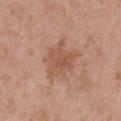Notes:
- biopsy status — catalogued during a skin exam; not biopsied
- diameter — about 5 mm
- anatomic site — the chest
- TBP lesion metrics — a shape eccentricity near 0.6 and two-axis asymmetry of about 0.35; an average lesion color of about L≈54 a*≈22 b*≈31 (CIELAB), a lesion–skin lightness drop of about 8, and a normalized lesion–skin contrast near 6; a color-variation rating of about 2.5/10 and radial color variation of about 1
- image source — total-body-photography crop, ~15 mm field of view
- lighting — white-light
- patient — female, aged approximately 40